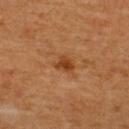Impression: Part of a total-body skin-imaging series; this lesion was reviewed on a skin check and was not flagged for biopsy. Image and clinical context: Imaged with cross-polarized lighting. Longest diameter approximately 2.5 mm. A male patient roughly 65 years of age. A lesion tile, about 15 mm wide, cut from a 3D total-body photograph. Automated image analysis of the tile measured a mean CIELAB color near L≈40 a*≈25 b*≈37 and about 10 CIELAB-L* units darker than the surrounding skin. The software also gave a color-variation rating of about 2.5/10. The analysis additionally found a nevus-likeness score of about 65/100 and lesion-presence confidence of about 100/100. On the back.